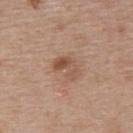Captured during whole-body skin photography for melanoma surveillance; the lesion was not biopsied. From the upper back. A female subject, about 40 years old. The recorded lesion diameter is about 3 mm. This is a white-light tile. Automated image analysis of the tile measured an outline eccentricity of about 0.8 (0 = round, 1 = elongated) and two-axis asymmetry of about 0.5. The software also gave a lesion–skin lightness drop of about 9 and a normalized border contrast of about 7. It also reported a border-irregularity index near 5/10, a color-variation rating of about 5.5/10, and radial color variation of about 2. A region of skin cropped from a whole-body photographic capture, roughly 15 mm wide.